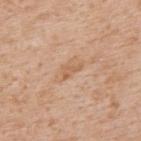No biopsy was performed on this lesion — it was imaged during a full skin examination and was not determined to be concerning. The tile uses white-light illumination. Approximately 2.5 mm at its widest. From the upper back. Cropped from a whole-body photographic skin survey; the tile spans about 15 mm. A male patient, aged around 65.